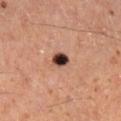Clinical impression:
Imaged during a routine full-body skin examination; the lesion was not biopsied and no histopathology is available.
Acquisition and patient details:
A 15 mm close-up extracted from a 3D total-body photography capture. Located on the right lower leg. Captured under cross-polarized illumination. The subject is a male aged around 50.